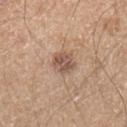<lesion>
  <biopsy_status>not biopsied; imaged during a skin examination</biopsy_status>
  <lighting>white-light</lighting>
  <automated_metrics>
    <eccentricity>0.5</eccentricity>
    <shape_asymmetry>0.2</shape_asymmetry>
    <vs_skin_darker_L>11.0</vs_skin_darker_L>
    <vs_skin_contrast_norm>7.5</vs_skin_contrast_norm>
    <border_irregularity_0_10>2.0</border_irregularity_0_10>
    <color_variation_0_10>3.5</color_variation_0_10>
    <peripheral_color_asymmetry>1.5</peripheral_color_asymmetry>
    <nevus_likeness_0_100>30</nevus_likeness_0_100>
    <lesion_detection_confidence_0_100>100</lesion_detection_confidence_0_100>
  </automated_metrics>
  <patient>
    <sex>male</sex>
    <age_approx>40</age_approx>
  </patient>
  <image>
    <source>total-body photography crop</source>
    <field_of_view_mm>15</field_of_view_mm>
  </image>
  <site>arm</site>
</lesion>